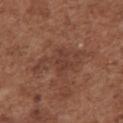This lesion was catalogued during total-body skin photography and was not selected for biopsy. The tile uses white-light illumination. The lesion is on the chest. A lesion tile, about 15 mm wide, cut from a 3D total-body photograph. Longest diameter approximately 6.5 mm. A female subject approximately 75 years of age.